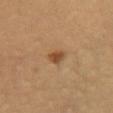The lesion was photographed on a routine skin check and not biopsied; there is no pathology result. Automated tile analysis of the lesion measured a footprint of about 3.5 mm², an eccentricity of roughly 0.5, and two-axis asymmetry of about 0.3. It also reported an average lesion color of about L≈45 a*≈19 b*≈34 (CIELAB), roughly 10 lightness units darker than nearby skin, and a normalized lesion–skin contrast near 8. The software also gave a border-irregularity rating of about 2.5/10 and a peripheral color-asymmetry measure near 1.5. On the chest. A female patient, in their 30s. Captured under cross-polarized illumination. The lesion's longest dimension is about 2 mm. Cropped from a total-body skin-imaging series; the visible field is about 15 mm.